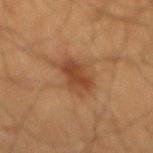follow-up: total-body-photography surveillance lesion; no biopsy | location: the mid back | patient: male, in their mid- to late 60s | size: ≈5 mm | lighting: cross-polarized | image: 15 mm crop, total-body photography.